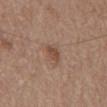Assessment:
No biopsy was performed on this lesion — it was imaged during a full skin examination and was not determined to be concerning.
Acquisition and patient details:
Imaged with white-light lighting. The patient is a male approximately 70 years of age. From the mid back. The total-body-photography lesion software estimated a footprint of about 3.5 mm² and a symmetry-axis asymmetry near 0.25. And it measured a mean CIELAB color near L≈48 a*≈19 b*≈28, a lesion–skin lightness drop of about 9, and a normalized lesion–skin contrast near 7. Cropped from a total-body skin-imaging series; the visible field is about 15 mm.